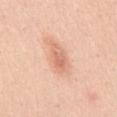notes — total-body-photography surveillance lesion; no biopsy
lighting — white-light
image — total-body-photography crop, ~15 mm field of view
site — the mid back
patient — female, aged 38–42